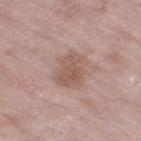Impression:
The lesion was tiled from a total-body skin photograph and was not biopsied.